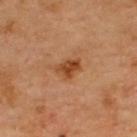Q: Was a biopsy performed?
A: no biopsy performed (imaged during a skin exam)
Q: Automated lesion metrics?
A: a lesion area of about 5 mm²; a border-irregularity rating of about 3/10, internal color variation of about 3.5 on a 0–10 scale, and a peripheral color-asymmetry measure near 1.5
Q: What lighting was used for the tile?
A: cross-polarized
Q: How was this image acquired?
A: total-body-photography crop, ~15 mm field of view
Q: Lesion size?
A: ~3 mm (longest diameter)
Q: Where on the body is the lesion?
A: the upper back
Q: Who is the patient?
A: male, roughly 70 years of age